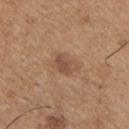follow-up — catalogued during a skin exam; not biopsied.